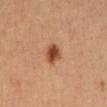workup: total-body-photography surveillance lesion; no biopsy
image source: ~15 mm crop, total-body skin-cancer survey
subject: male, aged 63–67
automated metrics: a lesion color around L≈47 a*≈24 b*≈35 in CIELAB, roughly 14 lightness units darker than nearby skin, and a normalized lesion–skin contrast near 10.5; a border-irregularity index near 1.5/10, a within-lesion color-variation index near 5.5/10, and peripheral color asymmetry of about 2; a detector confidence of about 100 out of 100 that the crop contains a lesion
body site: the abdomen
lesion diameter: about 2.5 mm
lighting: cross-polarized